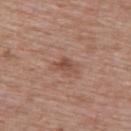Findings:
* notes — total-body-photography surveillance lesion; no biopsy
* site — the upper back
* patient — female, aged approximately 60
* diameter — about 2.5 mm
* lighting — white-light
* automated metrics — a footprint of about 3.5 mm², a shape eccentricity near 0.75, and a symmetry-axis asymmetry near 0.4; a lesion color around L≈49 a*≈21 b*≈27 in CIELAB, about 9 CIELAB-L* units darker than the surrounding skin, and a lesion-to-skin contrast of about 6.5 (normalized; higher = more distinct); a border-irregularity rating of about 4/10, a within-lesion color-variation index near 2/10, and a peripheral color-asymmetry measure near 0.5; a lesion-detection confidence of about 100/100
* acquisition — 15 mm crop, total-body photography